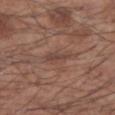Assessment:
Recorded during total-body skin imaging; not selected for excision or biopsy.
Acquisition and patient details:
A male subject, roughly 55 years of age. The lesion is located on the right forearm. This is a white-light tile. The lesion's longest dimension is about 2.5 mm. A region of skin cropped from a whole-body photographic capture, roughly 15 mm wide. Automated tile analysis of the lesion measured a lesion color around L≈43 a*≈18 b*≈24 in CIELAB, about 7 CIELAB-L* units darker than the surrounding skin, and a normalized lesion–skin contrast near 5.5. And it measured a border-irregularity rating of about 3.5/10, internal color variation of about 1 on a 0–10 scale, and radial color variation of about 0.5. And it measured a classifier nevus-likeness of about 0/100 and lesion-presence confidence of about 100/100.